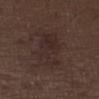Q: Was a biopsy performed?
A: catalogued during a skin exam; not biopsied
Q: What are the patient's age and sex?
A: male, roughly 70 years of age
Q: Where on the body is the lesion?
A: the leg
Q: How was this image acquired?
A: total-body-photography crop, ~15 mm field of view
Q: How was the tile lit?
A: white-light